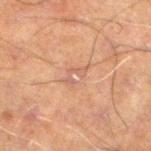{
  "biopsy_status": "not biopsied; imaged during a skin examination"
}